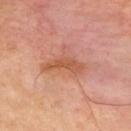{"biopsy_status": "not biopsied; imaged during a skin examination", "patient": {"sex": "male", "age_approx": 50}, "lesion_size": {"long_diameter_mm_approx": 5.0}, "lighting": "cross-polarized", "site": "back", "image": {"source": "total-body photography crop", "field_of_view_mm": 15}}